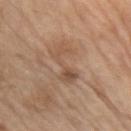No biopsy was performed on this lesion — it was imaged during a full skin examination and was not determined to be concerning.
From the right upper arm.
An algorithmic analysis of the crop reported a footprint of about 7 mm² and an eccentricity of roughly 0.95. The software also gave a lesion color around L≈52 a*≈18 b*≈30 in CIELAB, roughly 8 lightness units darker than nearby skin, and a normalized lesion–skin contrast near 6.
A male subject approximately 70 years of age.
A lesion tile, about 15 mm wide, cut from a 3D total-body photograph.
The lesion's longest dimension is about 5 mm.